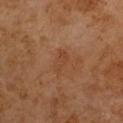{"image": {"source": "total-body photography crop", "field_of_view_mm": 15}, "patient": {"sex": "male", "age_approx": 65}, "automated_metrics": {"cielab_L": 42, "cielab_a": 22, "cielab_b": 32, "nevus_likeness_0_100": 0, "lesion_detection_confidence_0_100": 100}}